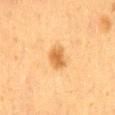Clinical impression:
The lesion was tiled from a total-body skin photograph and was not biopsied.
Context:
A male subject, aged 33–37. The total-body-photography lesion software estimated a mean CIELAB color near L≈51 a*≈19 b*≈38, about 9 CIELAB-L* units darker than the surrounding skin, and a normalized lesion–skin contrast near 7.5. It also reported a border-irregularity rating of about 2/10 and a color-variation rating of about 2.5/10. It also reported a detector confidence of about 100 out of 100 that the crop contains a lesion. A 15 mm crop from a total-body photograph taken for skin-cancer surveillance. Approximately 3 mm at its widest. From the mid back. The tile uses cross-polarized illumination.